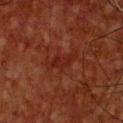Assessment:
No biopsy was performed on this lesion — it was imaged during a full skin examination and was not determined to be concerning.
Clinical summary:
A roughly 15 mm field-of-view crop from a total-body skin photograph. Longest diameter approximately 4 mm. The total-body-photography lesion software estimated an area of roughly 5 mm², an outline eccentricity of about 0.9 (0 = round, 1 = elongated), and a symmetry-axis asymmetry near 0.35. Imaged with cross-polarized lighting. On the chest. A male subject, roughly 65 years of age.